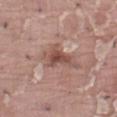<record>
  <biopsy_status>not biopsied; imaged during a skin examination</biopsy_status>
  <automated_metrics>
    <color_variation_0_10>5.0</color_variation_0_10>
    <peripheral_color_asymmetry>1.5</peripheral_color_asymmetry>
    <lesion_detection_confidence_0_100>95</lesion_detection_confidence_0_100>
  </automated_metrics>
  <patient>
    <sex>male</sex>
    <age_approx>40</age_approx>
  </patient>
  <image>
    <source>total-body photography crop</source>
    <field_of_view_mm>15</field_of_view_mm>
  </image>
  <site>abdomen</site>
  <lesion_size>
    <long_diameter_mm_approx>5.0</long_diameter_mm_approx>
  </lesion_size>
  <lighting>white-light</lighting>
</record>